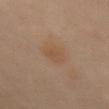Impression:
No biopsy was performed on this lesion — it was imaged during a full skin examination and was not determined to be concerning.
Context:
Automated image analysis of the tile measured an area of roughly 6 mm² and a shape eccentricity near 0.65. And it measured a mean CIELAB color near L≈43 a*≈15 b*≈27 and a normalized border contrast of about 4.5. A female subject aged around 60. Imaged with cross-polarized lighting. From the mid back. A region of skin cropped from a whole-body photographic capture, roughly 15 mm wide. About 3 mm across.